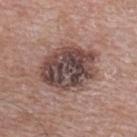Q: Is there a histopathology result?
A: no biopsy performed (imaged during a skin exam)
Q: What is the imaging modality?
A: ~15 mm crop, total-body skin-cancer survey
Q: Where on the body is the lesion?
A: the back
Q: Patient demographics?
A: male, in their mid- to late 60s